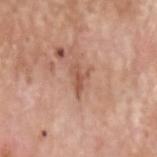follow-up = no biopsy performed (imaged during a skin exam)
imaging modality = ~15 mm crop, total-body skin-cancer survey
illumination = white-light
patient = male, aged approximately 80
automated lesion analysis = a lesion area of about 3 mm², a shape eccentricity near 0.85, and two-axis asymmetry of about 0.5; an average lesion color of about L≈54 a*≈23 b*≈31 (CIELAB), roughly 10 lightness units darker than nearby skin, and a normalized border contrast of about 6.5; an automated nevus-likeness rating near 0 out of 100
body site = the head or neck
size = ≈3 mm